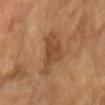biopsy status: imaged on a skin check; not biopsied | imaging modality: 15 mm crop, total-body photography | lighting: cross-polarized illumination | patient: male, aged 63 to 67.